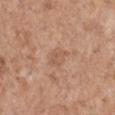Imaged during a routine full-body skin examination; the lesion was not biopsied and no histopathology is available.
The patient is a male in their 70s.
The lesion is on the right upper arm.
A close-up tile cropped from a whole-body skin photograph, about 15 mm across.